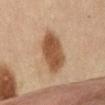follow-up: imaged on a skin check; not biopsied
imaging modality: 15 mm crop, total-body photography
anatomic site: the abdomen
lesion size: ~6 mm (longest diameter)
automated metrics: a mean CIELAB color near L≈45 a*≈18 b*≈30, about 13 CIELAB-L* units darker than the surrounding skin, and a lesion-to-skin contrast of about 10 (normalized; higher = more distinct); a border-irregularity rating of about 1.5/10 and peripheral color asymmetry of about 1; a nevus-likeness score of about 100/100 and a lesion-detection confidence of about 100/100
patient: female, approximately 60 years of age
tile lighting: cross-polarized